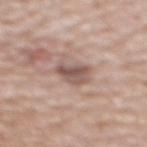Captured during whole-body skin photography for melanoma surveillance; the lesion was not biopsied. Longest diameter approximately 3.5 mm. A 15 mm close-up tile from a total-body photography series done for melanoma screening. The lesion is located on the upper back. Automated image analysis of the tile measured an area of roughly 6 mm², an eccentricity of roughly 0.7, and a symmetry-axis asymmetry near 0.3. And it measured a mean CIELAB color near L≈53 a*≈17 b*≈22, a lesion–skin lightness drop of about 12, and a normalized lesion–skin contrast near 8. A female patient in their 40s.